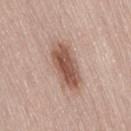The lesion was photographed on a routine skin check and not biopsied; there is no pathology result. The lesion is located on the leg. This is a white-light tile. The lesion-visualizer software estimated roughly 14 lightness units darker than nearby skin. The software also gave a within-lesion color-variation index near 4.5/10 and radial color variation of about 1.5. The software also gave a nevus-likeness score of about 95/100 and lesion-presence confidence of about 100/100. A close-up tile cropped from a whole-body skin photograph, about 15 mm across. The patient is a female aged 48 to 52. The recorded lesion diameter is about 5.5 mm.